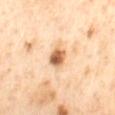<lesion>
<biopsy_status>not biopsied; imaged during a skin examination</biopsy_status>
<lesion_size>
  <long_diameter_mm_approx>2.5</long_diameter_mm_approx>
</lesion_size>
<site>mid back</site>
<lighting>cross-polarized</lighting>
<automated_metrics>
  <cielab_L>64</cielab_L>
  <cielab_a>23</cielab_a>
  <cielab_b>38</cielab_b>
  <vs_skin_darker_L>18.0</vs_skin_darker_L>
  <vs_skin_contrast_norm>10.5</vs_skin_contrast_norm>
  <border_irregularity_0_10>2.5</border_irregularity_0_10>
  <color_variation_0_10>6.0</color_variation_0_10>
  <peripheral_color_asymmetry>2.0</peripheral_color_asymmetry>
</automated_metrics>
<image>
  <source>total-body photography crop</source>
  <field_of_view_mm>15</field_of_view_mm>
</image>
<patient>
  <sex>male</sex>
  <age_approx>65</age_approx>
</patient>
</lesion>